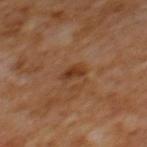Clinical impression: Imaged during a routine full-body skin examination; the lesion was not biopsied and no histopathology is available. Acquisition and patient details: On the mid back. A male patient aged 63 to 67. Longest diameter approximately 2.5 mm. Imaged with cross-polarized lighting. Cropped from a whole-body photographic skin survey; the tile spans about 15 mm.